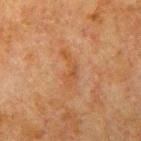Clinical impression:
The lesion was photographed on a routine skin check and not biopsied; there is no pathology result.
Image and clinical context:
A male subject aged approximately 80. The total-body-photography lesion software estimated an average lesion color of about L≈41 a*≈20 b*≈33 (CIELAB), about 5 CIELAB-L* units darker than the surrounding skin, and a normalized border contrast of about 5.5. About 4.5 mm across. Captured under cross-polarized illumination. Cropped from a total-body skin-imaging series; the visible field is about 15 mm. On the left upper arm.